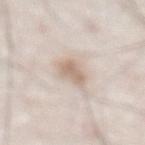Located on the abdomen.
Automated image analysis of the tile measured an average lesion color of about L≈67 a*≈14 b*≈25 (CIELAB) and about 11 CIELAB-L* units darker than the surrounding skin. The analysis additionally found an automated nevus-likeness rating near 65 out of 100.
A male subject about 80 years old.
A roughly 15 mm field-of-view crop from a total-body skin photograph.
About 3.5 mm across.